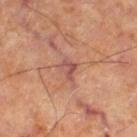Clinical impression:
Recorded during total-body skin imaging; not selected for excision or biopsy.
Clinical summary:
A 15 mm close-up extracted from a 3D total-body photography capture. The subject is approximately 65 years of age. From the right thigh.